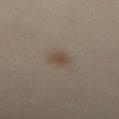Notes:
– follow-up — total-body-photography surveillance lesion; no biopsy
– image source — ~15 mm tile from a whole-body skin photo
– anatomic site — the abdomen
– image-analysis metrics — a lesion area of about 4.5 mm², an eccentricity of roughly 0.7, and a symmetry-axis asymmetry near 0.2; a mean CIELAB color near L≈47 a*≈11 b*≈25, roughly 7 lightness units darker than nearby skin, and a normalized border contrast of about 6.5; border irregularity of about 1.5 on a 0–10 scale, a within-lesion color-variation index near 2/10, and radial color variation of about 0.5
– subject — female, roughly 40 years of age